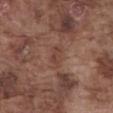{"biopsy_status": "not biopsied; imaged during a skin examination", "lesion_size": {"long_diameter_mm_approx": 3.0}, "patient": {"sex": "male", "age_approx": 75}, "automated_metrics": {"cielab_L": 42, "cielab_a": 20, "cielab_b": 25, "vs_skin_contrast_norm": 5.0, "border_irregularity_0_10": 3.0, "color_variation_0_10": 3.5, "peripheral_color_asymmetry": 1.0}, "image": {"source": "total-body photography crop", "field_of_view_mm": 15}, "lighting": "white-light", "site": "abdomen"}